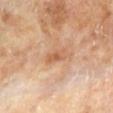Recorded during total-body skin imaging; not selected for excision or biopsy.
On the right leg.
A female subject aged 63–67.
A lesion tile, about 15 mm wide, cut from a 3D total-body photograph.